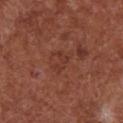Findings:
• illumination — white-light
• subject — female, approximately 75 years of age
• image source — 15 mm crop, total-body photography
• site — the chest
• automated lesion analysis — a lesion–skin lightness drop of about 5 and a normalized border contrast of about 4.5; internal color variation of about 0 on a 0–10 scale; a detector confidence of about 100 out of 100 that the crop contains a lesion